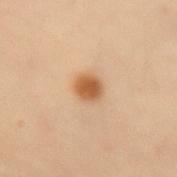Imaged during a routine full-body skin examination; the lesion was not biopsied and no histopathology is available.
The recorded lesion diameter is about 2.5 mm.
Cropped from a total-body skin-imaging series; the visible field is about 15 mm.
The lesion is on the left forearm.
The tile uses cross-polarized illumination.
A female subject, aged around 35.